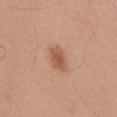  biopsy_status: not biopsied; imaged during a skin examination
  image:
    source: total-body photography crop
    field_of_view_mm: 15
  lesion_size:
    long_diameter_mm_approx: 3.5
  site: chest
  lighting: white-light
  patient:
    sex: male
    age_approx: 30